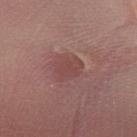This lesion was catalogued during total-body skin photography and was not selected for biopsy.
The lesion is located on the right forearm.
A region of skin cropped from a whole-body photographic capture, roughly 15 mm wide.
A female subject aged approximately 30.
Automated image analysis of the tile measured an average lesion color of about L≈45 a*≈23 b*≈21 (CIELAB), roughly 6 lightness units darker than nearby skin, and a normalized border contrast of about 5. It also reported a border-irregularity rating of about 2.5/10 and radial color variation of about 0.5. The software also gave a classifier nevus-likeness of about 0/100 and lesion-presence confidence of about 95/100.
This is a white-light tile.
Longest diameter approximately 3 mm.